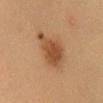Clinical impression: The lesion was photographed on a routine skin check and not biopsied; there is no pathology result. Image and clinical context: About 5 mm across. A 15 mm close-up tile from a total-body photography series done for melanoma screening. The tile uses cross-polarized illumination. A female patient, in their 30s. Automated tile analysis of the lesion measured a lesion area of about 13 mm², an outline eccentricity of about 0.75 (0 = round, 1 = elongated), and a symmetry-axis asymmetry near 0.35. And it measured border irregularity of about 3.5 on a 0–10 scale and internal color variation of about 3.5 on a 0–10 scale. And it measured an automated nevus-likeness rating near 95 out of 100. The lesion is on the head or neck.